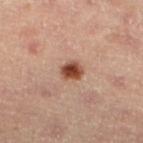Assessment: This lesion was catalogued during total-body skin photography and was not selected for biopsy. Clinical summary: Captured under cross-polarized illumination. Automated image analysis of the tile measured a lesion area of about 5 mm² and two-axis asymmetry of about 0.25. And it measured a detector confidence of about 100 out of 100 that the crop contains a lesion. The patient is a female aged 43–47. The lesion is located on the left lower leg. Cropped from a whole-body photographic skin survey; the tile spans about 15 mm.